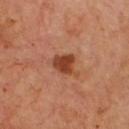The lesion was tiled from a total-body skin photograph and was not biopsied. The tile uses cross-polarized illumination. The lesion is located on the chest. A lesion tile, about 15 mm wide, cut from a 3D total-body photograph. The subject is a male aged around 65.